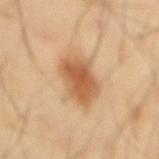biopsy_status: not biopsied; imaged during a skin examination
lighting: cross-polarized
patient:
  sex: male
  age_approx: 40
site: mid back
image:
  source: total-body photography crop
  field_of_view_mm: 15